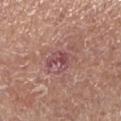| feature | finding |
|---|---|
| lesion size | ~4 mm (longest diameter) |
| patient | male, aged around 75 |
| illumination | white-light |
| image | ~15 mm crop, total-body skin-cancer survey |
| location | the left lower leg |
| TBP lesion metrics | an average lesion color of about L≈49 a*≈24 b*≈19 (CIELAB), about 9 CIELAB-L* units darker than the surrounding skin, and a normalized lesion–skin contrast near 7.5 |